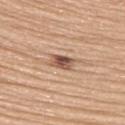notes = imaged on a skin check; not biopsied | illumination = white-light | anatomic site = the upper back | patient = female, approximately 65 years of age | lesion diameter = ~3 mm (longest diameter) | image = ~15 mm tile from a whole-body skin photo.